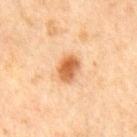Assessment:
Imaged during a routine full-body skin examination; the lesion was not biopsied and no histopathology is available.
Context:
Captured under cross-polarized illumination. The lesion's longest dimension is about 3 mm. A 15 mm close-up extracted from a 3D total-body photography capture. A male subject in their mid-60s. From the chest. The total-body-photography lesion software estimated a mean CIELAB color near L≈54 a*≈23 b*≈37, roughly 13 lightness units darker than nearby skin, and a normalized border contrast of about 9.5. It also reported an automated nevus-likeness rating near 100 out of 100.